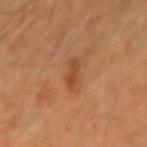  biopsy_status: not biopsied; imaged during a skin examination